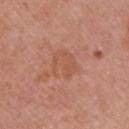Q: Was this lesion biopsied?
A: total-body-photography surveillance lesion; no biopsy
Q: Lesion size?
A: about 3.5 mm
Q: What kind of image is this?
A: ~15 mm crop, total-body skin-cancer survey
Q: Who is the patient?
A: female, aged 38–42
Q: Lesion location?
A: the right upper arm
Q: Illumination type?
A: white-light illumination